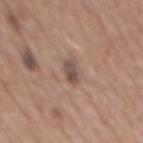This lesion was catalogued during total-body skin photography and was not selected for biopsy.
The lesion is on the mid back.
The tile uses white-light illumination.
A lesion tile, about 15 mm wide, cut from a 3D total-body photograph.
Automated tile analysis of the lesion measured an automated nevus-likeness rating near 10 out of 100 and a lesion-detection confidence of about 100/100.
The lesion's longest dimension is about 3 mm.
The patient is a male aged approximately 65.